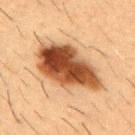biopsy status — catalogued during a skin exam; not biopsied | automated metrics — a nevus-likeness score of about 85/100 and a detector confidence of about 100 out of 100 that the crop contains a lesion | lesion diameter — ≈9 mm | image source — total-body-photography crop, ~15 mm field of view | anatomic site — the chest | subject — male, aged approximately 55 | illumination — cross-polarized illumination.